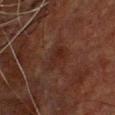Imaged during a routine full-body skin examination; the lesion was not biopsied and no histopathology is available. A roughly 15 mm field-of-view crop from a total-body skin photograph. Located on the chest. The patient is a male aged approximately 65.